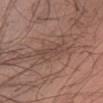The lesion was tiled from a total-body skin photograph and was not biopsied.